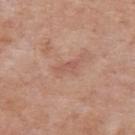Findings:
* biopsy status · no biopsy performed (imaged during a skin exam)
* patient · female, roughly 60 years of age
* size · about 3 mm
* site · the upper back
* image source · ~15 mm crop, total-body skin-cancer survey
* lighting · white-light illumination